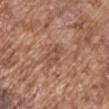The recorded lesion diameter is about 2.5 mm. Located on the right upper arm. Automated tile analysis of the lesion measured a footprint of about 3.5 mm² and a symmetry-axis asymmetry near 0.4. The analysis additionally found an average lesion color of about L≈49 a*≈21 b*≈29 (CIELAB), roughly 7 lightness units darker than nearby skin, and a normalized border contrast of about 5.5. The software also gave peripheral color asymmetry of about 1. A region of skin cropped from a whole-body photographic capture, roughly 15 mm wide. A male subject, in their mid- to late 70s. Captured under white-light illumination.